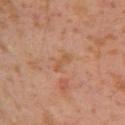Q: Was this lesion biopsied?
A: imaged on a skin check; not biopsied
Q: What are the patient's age and sex?
A: male, aged around 30
Q: Lesion location?
A: the left arm
Q: What kind of image is this?
A: total-body-photography crop, ~15 mm field of view
Q: Illumination type?
A: cross-polarized
Q: What is the lesion's diameter?
A: ~3 mm (longest diameter)
Q: Automated lesion metrics?
A: an area of roughly 3 mm², an outline eccentricity of about 0.9 (0 = round, 1 = elongated), and a symmetry-axis asymmetry near 0.3; a classifier nevus-likeness of about 0/100 and lesion-presence confidence of about 100/100